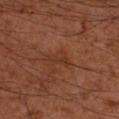This lesion was catalogued during total-body skin photography and was not selected for biopsy. The tile uses cross-polarized illumination. A 15 mm close-up tile from a total-body photography series done for melanoma screening. Longest diameter approximately 3 mm. Automated tile analysis of the lesion measured an outline eccentricity of about 0.8 (0 = round, 1 = elongated) and two-axis asymmetry of about 0.45. And it measured a mean CIELAB color near L≈35 a*≈23 b*≈31, roughly 5 lightness units darker than nearby skin, and a normalized lesion–skin contrast near 5. And it measured a nevus-likeness score of about 0/100 and a lesion-detection confidence of about 100/100. The subject is a male roughly 55 years of age. On the right upper arm.